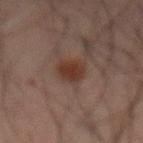<lesion>
  <biopsy_status>not biopsied; imaged during a skin examination</biopsy_status>
  <patient>
    <sex>male</sex>
    <age_approx>70</age_approx>
  </patient>
  <lighting>cross-polarized</lighting>
  <image>
    <source>total-body photography crop</source>
    <field_of_view_mm>15</field_of_view_mm>
  </image>
  <site>lower back</site>
  <lesion_size>
    <long_diameter_mm_approx>3.5</long_diameter_mm_approx>
  </lesion_size>
</lesion>